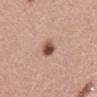{"biopsy_status": "not biopsied; imaged during a skin examination", "lesion_size": {"long_diameter_mm_approx": 3.0}, "lighting": "white-light", "automated_metrics": {"area_mm2_approx": 4.0, "eccentricity": 0.8, "shape_asymmetry": 0.25, "border_irregularity_0_10": 2.5, "color_variation_0_10": 6.5, "peripheral_color_asymmetry": 2.0}, "image": {"source": "total-body photography crop", "field_of_view_mm": 15}, "patient": {"sex": "male", "age_approx": 55}, "site": "chest"}